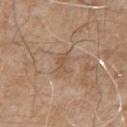Impression: The lesion was tiled from a total-body skin photograph and was not biopsied. Image and clinical context: A male subject, about 55 years old. From the chest. Approximately 3 mm at its widest. A 15 mm close-up extracted from a 3D total-body photography capture. Captured under white-light illumination.